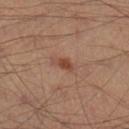workup: imaged on a skin check; not biopsied | illumination: cross-polarized illumination | body site: the right lower leg | TBP lesion metrics: a footprint of about 2.5 mm², an eccentricity of roughly 0.85, and a shape-asymmetry score of about 0.35 (0 = symmetric); about 8 CIELAB-L* units darker than the surrounding skin and a normalized border contrast of about 7.5; a peripheral color-asymmetry measure near 0.5 | imaging modality: ~15 mm crop, total-body skin-cancer survey | size: ≈2.5 mm | subject: male, aged approximately 40.